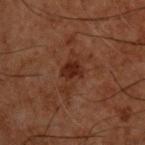Clinical impression: Imaged during a routine full-body skin examination; the lesion was not biopsied and no histopathology is available. Context: The tile uses cross-polarized illumination. From the upper back. Automated tile analysis of the lesion measured a lesion area of about 10 mm², an eccentricity of roughly 0.9, and a shape-asymmetry score of about 0.4 (0 = symmetric). The software also gave a lesion color around L≈22 a*≈18 b*≈22 in CIELAB, a lesion–skin lightness drop of about 5, and a normalized border contrast of about 6.5. The software also gave border irregularity of about 5.5 on a 0–10 scale and peripheral color asymmetry of about 0.5. And it measured a nevus-likeness score of about 45/100 and a detector confidence of about 100 out of 100 that the crop contains a lesion. A male subject roughly 50 years of age. About 5.5 mm across. A roughly 15 mm field-of-view crop from a total-body skin photograph.